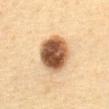workup=no biopsy performed (imaged during a skin exam)
automated lesion analysis=two-axis asymmetry of about 0.1; a mean CIELAB color near L≈46 a*≈18 b*≈30 and a lesion–skin lightness drop of about 21
site=the abdomen
acquisition=~15 mm crop, total-body skin-cancer survey
patient=female, roughly 50 years of age
size=~5 mm (longest diameter)